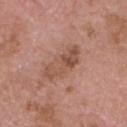workup = catalogued during a skin exam; not biopsied | size = ~5 mm (longest diameter) | acquisition = 15 mm crop, total-body photography | patient = male, aged around 75 | image-analysis metrics = a lesion area of about 8 mm², a shape eccentricity near 0.9, and two-axis asymmetry of about 0.45; an average lesion color of about L≈51 a*≈22 b*≈28 (CIELAB), about 9 CIELAB-L* units darker than the surrounding skin, and a normalized border contrast of about 6.5 | body site = the head or neck | tile lighting = white-light.